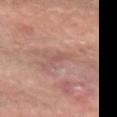Impression: No biopsy was performed on this lesion — it was imaged during a full skin examination and was not determined to be concerning. Clinical summary: The lesion is on the left forearm. This is a cross-polarized tile. A male subject in their mid- to late 50s. Approximately 1 mm at its widest. A 15 mm close-up tile from a total-body photography series done for melanoma screening. Automated image analysis of the tile measured a normalized border contrast of about 4. It also reported border irregularity of about 3.5 on a 0–10 scale, internal color variation of about 0 on a 0–10 scale, and radial color variation of about 0. It also reported a classifier nevus-likeness of about 0/100 and lesion-presence confidence of about 60/100.